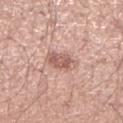Q: Was a biopsy performed?
A: total-body-photography surveillance lesion; no biopsy
Q: What did automated image analysis measure?
A: a footprint of about 6.5 mm², a shape eccentricity near 0.8, and a shape-asymmetry score of about 0.2 (0 = symmetric)
Q: Patient demographics?
A: male, approximately 45 years of age
Q: What is the anatomic site?
A: the right lower leg
Q: What is the imaging modality?
A: ~15 mm tile from a whole-body skin photo
Q: How was the tile lit?
A: white-light
Q: What is the lesion's diameter?
A: ~3.5 mm (longest diameter)